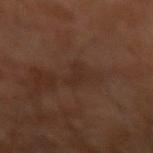size: about 2.5 mm | patient: male, aged approximately 60 | tile lighting: cross-polarized | imaging modality: 15 mm crop, total-body photography | anatomic site: the left forearm | image-analysis metrics: a footprint of about 3.5 mm² and an eccentricity of roughly 0.85; a mean CIELAB color near L≈25 a*≈16 b*≈21, roughly 4 lightness units darker than nearby skin, and a lesion-to-skin contrast of about 4.5 (normalized; higher = more distinct); a border-irregularity rating of about 4.5/10, internal color variation of about 0.5 on a 0–10 scale, and radial color variation of about 0.